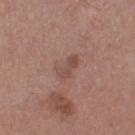{
  "biopsy_status": "not biopsied; imaged during a skin examination",
  "image": {
    "source": "total-body photography crop",
    "field_of_view_mm": 15
  },
  "automated_metrics": {
    "area_mm2_approx": 5.0,
    "eccentricity": 0.85,
    "shape_asymmetry": 0.25,
    "cielab_L": 48,
    "cielab_a": 20,
    "cielab_b": 24,
    "vs_skin_darker_L": 7.0,
    "vs_skin_contrast_norm": 5.5
  },
  "site": "right thigh",
  "patient": {
    "sex": "male",
    "age_approx": 75
  },
  "lesion_size": {
    "long_diameter_mm_approx": 3.5
  },
  "lighting": "white-light"
}